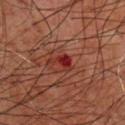Impression: This lesion was catalogued during total-body skin photography and was not selected for biopsy. Background: On the upper back. The patient is a male approximately 60 years of age. Cropped from a whole-body photographic skin survey; the tile spans about 15 mm. An algorithmic analysis of the crop reported a footprint of about 4 mm². The analysis additionally found a lesion color around L≈28 a*≈31 b*≈26 in CIELAB and about 9 CIELAB-L* units darker than the surrounding skin. The software also gave a border-irregularity index near 6.5/10, a color-variation rating of about 3/10, and peripheral color asymmetry of about 0.5. Measured at roughly 3 mm in maximum diameter.